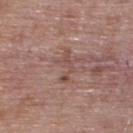No biopsy was performed on this lesion — it was imaged during a full skin examination and was not determined to be concerning. Cropped from a total-body skin-imaging series; the visible field is about 15 mm. The subject is a male about 65 years old. On the upper back. Approximately 4 mm at its widest.